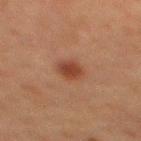The lesion was tiled from a total-body skin photograph and was not biopsied. A male subject, aged 58–62. The lesion is located on the upper back. Approximately 2.5 mm at its widest. A region of skin cropped from a whole-body photographic capture, roughly 15 mm wide.